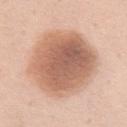Imaged during a routine full-body skin examination; the lesion was not biopsied and no histopathology is available. A female patient, in their 50s. The lesion is on the front of the torso. The lesion's longest dimension is about 9 mm. A close-up tile cropped from a whole-body skin photograph, about 15 mm across.